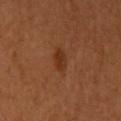Assessment:
This lesion was catalogued during total-body skin photography and was not selected for biopsy.
Clinical summary:
A 15 mm crop from a total-body photograph taken for skin-cancer surveillance. Longest diameter approximately 2.5 mm. On the left upper arm. This is a cross-polarized tile. A female subject, approximately 50 years of age.